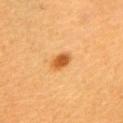Impression:
Part of a total-body skin-imaging series; this lesion was reviewed on a skin check and was not flagged for biopsy.
Context:
Automated image analysis of the tile measured a lesion color around L≈54 a*≈27 b*≈46 in CIELAB and roughly 13 lightness units darker than nearby skin. It also reported a border-irregularity index near 2/10, internal color variation of about 3 on a 0–10 scale, and a peripheral color-asymmetry measure near 1. A lesion tile, about 15 mm wide, cut from a 3D total-body photograph. A female subject, in their 30s. The tile uses cross-polarized illumination. Located on the chest.